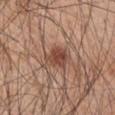<case>
<biopsy_status>not biopsied; imaged during a skin examination</biopsy_status>
<lesion_size>
  <long_diameter_mm_approx>4.0</long_diameter_mm_approx>
</lesion_size>
<automated_metrics>
  <area_mm2_approx>7.5</area_mm2_approx>
  <eccentricity>0.7</eccentricity>
  <shape_asymmetry>0.2</shape_asymmetry>
  <cielab_L>47</cielab_L>
  <cielab_a>21</cielab_a>
  <cielab_b>28</cielab_b>
  <vs_skin_darker_L>11.0</vs_skin_darker_L>
  <vs_skin_contrast_norm>8.0</vs_skin_contrast_norm>
  <border_irregularity_0_10>2.5</border_irregularity_0_10>
  <color_variation_0_10>4.0</color_variation_0_10>
  <peripheral_color_asymmetry>1.5</peripheral_color_asymmetry>
  <lesion_detection_confidence_0_100>100</lesion_detection_confidence_0_100>
</automated_metrics>
<site>left upper arm</site>
<image>
  <source>total-body photography crop</source>
  <field_of_view_mm>15</field_of_view_mm>
</image>
<lighting>white-light</lighting>
<patient>
  <sex>male</sex>
  <age_approx>45</age_approx>
</patient>
</case>